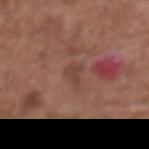The lesion was tiled from a total-body skin photograph and was not biopsied.
A region of skin cropped from a whole-body photographic capture, roughly 15 mm wide.
The patient is a female approximately 75 years of age.
Measured at roughly 2.5 mm in maximum diameter.
The tile uses white-light illumination.
From the back.
Automated image analysis of the tile measured a lesion area of about 3 mm², an outline eccentricity of about 0.7 (0 = round, 1 = elongated), and a shape-asymmetry score of about 0.45 (0 = symmetric). The software also gave a mean CIELAB color near L≈42 a*≈21 b*≈25, roughly 6 lightness units darker than nearby skin, and a normalized border contrast of about 5.5. And it measured lesion-presence confidence of about 100/100.